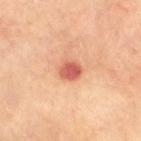An algorithmic analysis of the crop reported a nevus-likeness score of about 20/100 and a lesion-detection confidence of about 100/100.
Cropped from a total-body skin-imaging series; the visible field is about 15 mm.
A male subject, aged 63 to 67.
About 2.5 mm across.